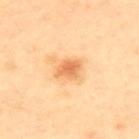Imaged during a routine full-body skin examination; the lesion was not biopsied and no histopathology is available. A lesion tile, about 15 mm wide, cut from a 3D total-body photograph. The lesion is located on the upper back. The recorded lesion diameter is about 3 mm. The subject is a female approximately 40 years of age. Imaged with cross-polarized lighting.